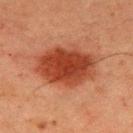No biopsy was performed on this lesion — it was imaged during a full skin examination and was not determined to be concerning. Captured under cross-polarized illumination. The lesion's longest dimension is about 7 mm. The subject is a male aged 58–62. The total-body-photography lesion software estimated a mean CIELAB color near L≈34 a*≈26 b*≈29, a lesion–skin lightness drop of about 11, and a normalized border contrast of about 10. It also reported a classifier nevus-likeness of about 100/100 and a detector confidence of about 100 out of 100 that the crop contains a lesion. Located on the upper back. Cropped from a total-body skin-imaging series; the visible field is about 15 mm.